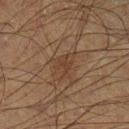Imaged during a routine full-body skin examination; the lesion was not biopsied and no histopathology is available. A lesion tile, about 15 mm wide, cut from a 3D total-body photograph. From the left lower leg. The tile uses cross-polarized illumination. Measured at roughly 3 mm in maximum diameter. A male subject, in their 50s.